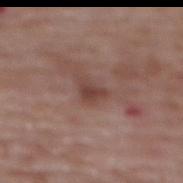Q: Lesion location?
A: the upper back
Q: Patient demographics?
A: female, in their mid-60s
Q: What did automated image analysis measure?
A: a footprint of about 3.5 mm², an eccentricity of roughly 0.65, and two-axis asymmetry of about 0.45; roughly 9 lightness units darker than nearby skin and a normalized border contrast of about 7; a color-variation rating of about 1.5/10 and radial color variation of about 0.5; an automated nevus-likeness rating near 0 out of 100 and a lesion-detection confidence of about 100/100
Q: What is the imaging modality?
A: ~15 mm crop, total-body skin-cancer survey
Q: What lighting was used for the tile?
A: white-light